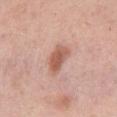Q: Was this lesion biopsied?
A: imaged on a skin check; not biopsied
Q: Who is the patient?
A: female, approximately 50 years of age
Q: Lesion size?
A: ~4 mm (longest diameter)
Q: What is the imaging modality?
A: ~15 mm crop, total-body skin-cancer survey
Q: How was the tile lit?
A: white-light illumination
Q: What did automated image analysis measure?
A: a mean CIELAB color near L≈58 a*≈23 b*≈29 and a normalized border contrast of about 8; a classifier nevus-likeness of about 85/100 and a lesion-detection confidence of about 100/100
Q: Where on the body is the lesion?
A: the right thigh